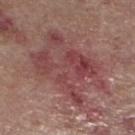Q: Was this lesion biopsied?
A: catalogued during a skin exam; not biopsied
Q: Lesion size?
A: ~9.5 mm (longest diameter)
Q: Who is the patient?
A: female, in their mid- to late 60s
Q: Lesion location?
A: the left lower leg
Q: How was the tile lit?
A: white-light
Q: What kind of image is this?
A: 15 mm crop, total-body photography